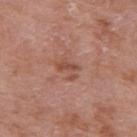follow-up: catalogued during a skin exam; not biopsied | image source: total-body-photography crop, ~15 mm field of view | tile lighting: white-light illumination | size: ≈3 mm | anatomic site: the right upper arm | subject: male, aged 68 to 72 | image-analysis metrics: an average lesion color of about L≈49 a*≈23 b*≈29 (CIELAB), a lesion–skin lightness drop of about 8, and a lesion-to-skin contrast of about 6.5 (normalized; higher = more distinct); a nevus-likeness score of about 0/100 and lesion-presence confidence of about 100/100.